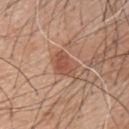Imaged during a routine full-body skin examination; the lesion was not biopsied and no histopathology is available. A 15 mm crop from a total-body photograph taken for skin-cancer surveillance. Imaged with white-light lighting. The lesion's longest dimension is about 3.5 mm. The lesion is located on the upper back. Automated tile analysis of the lesion measured an average lesion color of about L≈52 a*≈24 b*≈30 (CIELAB), about 9 CIELAB-L* units darker than the surrounding skin, and a lesion-to-skin contrast of about 7 (normalized; higher = more distinct). It also reported a classifier nevus-likeness of about 95/100 and a detector confidence of about 100 out of 100 that the crop contains a lesion. A male patient aged 48–52.